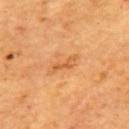biopsy status: no biopsy performed (imaged during a skin exam)
patient: male, aged approximately 65
TBP lesion metrics: a lesion area of about 3 mm², an eccentricity of roughly 0.95, and a shape-asymmetry score of about 0.5 (0 = symmetric); a border-irregularity rating of about 6/10, a color-variation rating of about 0/10, and a peripheral color-asymmetry measure near 0
size: ≈3.5 mm
anatomic site: the mid back
acquisition: 15 mm crop, total-body photography